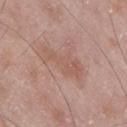Findings:
• follow-up — catalogued during a skin exam; not biopsied
• image source — 15 mm crop, total-body photography
• TBP lesion metrics — peripheral color asymmetry of about 0.5
• location — the right thigh
• illumination — white-light
• subject — male, aged approximately 50